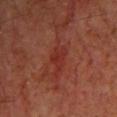notes: catalogued during a skin exam; not biopsied | image-analysis metrics: a lesion area of about 5 mm², an eccentricity of roughly 0.85, and a shape-asymmetry score of about 0.5 (0 = symmetric); a lesion–skin lightness drop of about 5 and a lesion-to-skin contrast of about 5 (normalized; higher = more distinct) | image source: ~15 mm tile from a whole-body skin photo | body site: the head or neck | diameter: ~4 mm (longest diameter) | subject: male, aged 58–62.